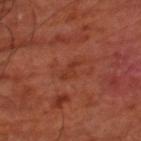The lesion was photographed on a routine skin check and not biopsied; there is no pathology result. The tile uses cross-polarized illumination. A patient aged 63–67. From the right lower leg. Cropped from a whole-body photographic skin survey; the tile spans about 15 mm.